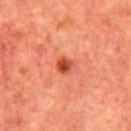biopsy status: no biopsy performed (imaged during a skin exam)
acquisition: ~15 mm tile from a whole-body skin photo
diameter: about 2 mm
location: the chest
patient: male, in their mid-60s
tile lighting: cross-polarized illumination